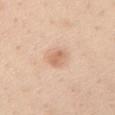| feature | finding |
|---|---|
| follow-up | no biopsy performed (imaged during a skin exam) |
| patient | male, aged 48 to 52 |
| imaging modality | total-body-photography crop, ~15 mm field of view |
| location | the chest |